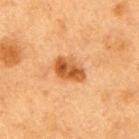follow-up — catalogued during a skin exam; not biopsied | anatomic site — the right upper arm | patient — female, aged 38–42 | imaging modality — ~15 mm tile from a whole-body skin photo | TBP lesion metrics — a footprint of about 8 mm²; border irregularity of about 2 on a 0–10 scale, a within-lesion color-variation index near 5.5/10, and peripheral color asymmetry of about 1.5; a detector confidence of about 100 out of 100 that the crop contains a lesion.